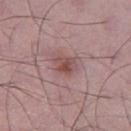Assessment: Captured during whole-body skin photography for melanoma surveillance; the lesion was not biopsied. Context: About 3 mm across. Captured under white-light illumination. A close-up tile cropped from a whole-body skin photograph, about 15 mm across. On the left lower leg. A male patient, roughly 50 years of age. An algorithmic analysis of the crop reported an area of roughly 5 mm², a shape eccentricity near 0.65, and a symmetry-axis asymmetry near 0.25. The analysis additionally found an average lesion color of about L≈49 a*≈21 b*≈21 (CIELAB), about 9 CIELAB-L* units darker than the surrounding skin, and a lesion-to-skin contrast of about 7.5 (normalized; higher = more distinct). The software also gave border irregularity of about 2.5 on a 0–10 scale and a color-variation rating of about 4.5/10.